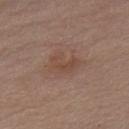<record>
<biopsy_status>not biopsied; imaged during a skin examination</biopsy_status>
<image>
  <source>total-body photography crop</source>
  <field_of_view_mm>15</field_of_view_mm>
</image>
<automated_metrics>
  <area_mm2_approx>4.5</area_mm2_approx>
  <nevus_likeness_0_100>0</nevus_likeness_0_100>
  <lesion_detection_confidence_0_100>100</lesion_detection_confidence_0_100>
</automated_metrics>
<lighting>white-light</lighting>
<site>front of the torso</site>
<lesion_size>
  <long_diameter_mm_approx>3.5</long_diameter_mm_approx>
</lesion_size>
<patient>
  <sex>male</sex>
  <age_approx>70</age_approx>
</patient>
</record>